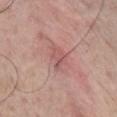Case summary:
– biopsy status — imaged on a skin check; not biopsied
– subject — male, in their mid-50s
– automated lesion analysis — an area of roughly 3.5 mm², a shape eccentricity near 0.85, and a shape-asymmetry score of about 0.45 (0 = symmetric); a lesion color around L≈55 a*≈25 b*≈22 in CIELAB, about 8 CIELAB-L* units darker than the surrounding skin, and a lesion-to-skin contrast of about 5.5 (normalized; higher = more distinct); a border-irregularity index near 4.5/10 and peripheral color asymmetry of about 1
– lighting — white-light
– location — the chest
– image source — total-body-photography crop, ~15 mm field of view
– lesion size — ≈3 mm